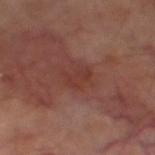<case>
  <biopsy_status>not biopsied; imaged during a skin examination</biopsy_status>
  <site>left thigh</site>
  <image>
    <source>total-body photography crop</source>
    <field_of_view_mm>15</field_of_view_mm>
  </image>
  <lighting>cross-polarized</lighting>
  <patient>
    <sex>male</sex>
    <age_approx>70</age_approx>
  </patient>
</case>